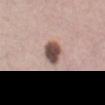Impression:
Part of a total-body skin-imaging series; this lesion was reviewed on a skin check and was not flagged for biopsy.
Image and clinical context:
A female patient in their mid- to late 30s. Imaged with white-light lighting. A 15 mm close-up tile from a total-body photography series done for melanoma screening. The lesion is located on the mid back.